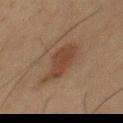Impression:
No biopsy was performed on this lesion — it was imaged during a full skin examination and was not determined to be concerning.
Clinical summary:
The lesion is on the chest. A region of skin cropped from a whole-body photographic capture, roughly 15 mm wide. The subject is a male in their 50s. Imaged with cross-polarized lighting. The recorded lesion diameter is about 5 mm.